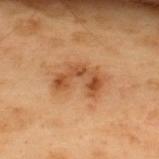The lesion was tiled from a total-body skin photograph and was not biopsied.
From the back.
A 15 mm crop from a total-body photograph taken for skin-cancer surveillance.
A male patient, aged approximately 55.